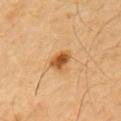| feature | finding |
|---|---|
| notes | total-body-photography surveillance lesion; no biopsy |
| anatomic site | the left upper arm |
| subject | male, in their mid-50s |
| acquisition | 15 mm crop, total-body photography |